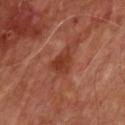Imaged during a routine full-body skin examination; the lesion was not biopsied and no histopathology is available.
The tile uses cross-polarized illumination.
A close-up tile cropped from a whole-body skin photograph, about 15 mm across.
A male subject, roughly 65 years of age.
Automated image analysis of the tile measured a lesion area of about 6.5 mm², a shape eccentricity near 0.8, and a shape-asymmetry score of about 0.4 (0 = symmetric). It also reported a border-irregularity rating of about 4/10 and a within-lesion color-variation index near 2.5/10. It also reported a classifier nevus-likeness of about 0/100.